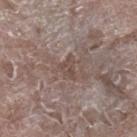Assessment:
Captured during whole-body skin photography for melanoma surveillance; the lesion was not biopsied.
Background:
A roughly 15 mm field-of-view crop from a total-body skin photograph. The recorded lesion diameter is about 3 mm. The subject is a male roughly 50 years of age. From the right lower leg. Imaged with white-light lighting. The lesion-visualizer software estimated two-axis asymmetry of about 0.45.